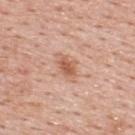The lesion was photographed on a routine skin check and not biopsied; there is no pathology result. The recorded lesion diameter is about 2.5 mm. From the upper back. This image is a 15 mm lesion crop taken from a total-body photograph. A male patient about 55 years old.